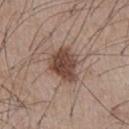follow-up = no biopsy performed (imaged during a skin exam)
imaging modality = ~15 mm crop, total-body skin-cancer survey
illumination = white-light
anatomic site = the chest
size = about 4 mm
automated metrics = a shape eccentricity near 0.6 and two-axis asymmetry of about 0.3; an automated nevus-likeness rating near 85 out of 100 and lesion-presence confidence of about 100/100
patient = male, in their mid- to late 50s